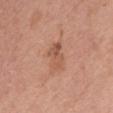Captured during whole-body skin photography for melanoma surveillance; the lesion was not biopsied.
Measured at roughly 3.5 mm in maximum diameter.
This image is a 15 mm lesion crop taken from a total-body photograph.
From the front of the torso.
The lesion-visualizer software estimated a lesion area of about 5.5 mm², a shape eccentricity near 0.85, and a symmetry-axis asymmetry near 0.35. The analysis additionally found a border-irregularity rating of about 4/10 and a peripheral color-asymmetry measure near 2.
A female patient aged approximately 45.